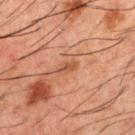This is a cross-polarized tile. A male subject, aged around 50. The lesion's longest dimension is about 2.5 mm. A 15 mm crop from a total-body photograph taken for skin-cancer surveillance. Located on the mid back.